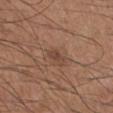Impression:
No biopsy was performed on this lesion — it was imaged during a full skin examination and was not determined to be concerning.
Image and clinical context:
Located on the leg. Longest diameter approximately 2.5 mm. A male subject, aged around 55. Cropped from a total-body skin-imaging series; the visible field is about 15 mm. The lesion-visualizer software estimated an area of roughly 3 mm², an eccentricity of roughly 0.85, and a symmetry-axis asymmetry near 0.35. It also reported an average lesion color of about L≈44 a*≈19 b*≈27 (CIELAB), about 8 CIELAB-L* units darker than the surrounding skin, and a normalized border contrast of about 6.5. And it measured a border-irregularity rating of about 3.5/10 and a peripheral color-asymmetry measure near 0. This is a white-light tile.